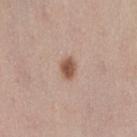Q: Is there a histopathology result?
A: catalogued during a skin exam; not biopsied
Q: Patient demographics?
A: female, aged around 40
Q: What did automated image analysis measure?
A: an area of roughly 4.5 mm²; a classifier nevus-likeness of about 100/100 and lesion-presence confidence of about 100/100
Q: Where on the body is the lesion?
A: the right thigh
Q: What is the imaging modality?
A: total-body-photography crop, ~15 mm field of view